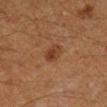Q: Where on the body is the lesion?
A: the right lower leg
Q: What kind of image is this?
A: ~15 mm tile from a whole-body skin photo
Q: What did automated image analysis measure?
A: a shape-asymmetry score of about 0.2 (0 = symmetric); a lesion color around L≈36 a*≈22 b*≈30 in CIELAB, roughly 8 lightness units darker than nearby skin, and a lesion-to-skin contrast of about 7 (normalized; higher = more distinct); border irregularity of about 1.5 on a 0–10 scale and a color-variation rating of about 3/10; an automated nevus-likeness rating near 70 out of 100 and a detector confidence of about 100 out of 100 that the crop contains a lesion
Q: What lighting was used for the tile?
A: cross-polarized illumination
Q: Who is the patient?
A: male, aged approximately 60